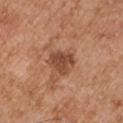Recorded during total-body skin imaging; not selected for excision or biopsy. The total-body-photography lesion software estimated an average lesion color of about L≈47 a*≈24 b*≈31 (CIELAB) and a lesion-to-skin contrast of about 8 (normalized; higher = more distinct). The software also gave a classifier nevus-likeness of about 15/100 and lesion-presence confidence of about 100/100. About 3.5 mm across. A male patient, aged around 55. Captured under white-light illumination. On the right upper arm. This image is a 15 mm lesion crop taken from a total-body photograph.